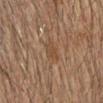Q: Was this lesion biopsied?
A: catalogued during a skin exam; not biopsied
Q: What are the patient's age and sex?
A: male, in their mid- to late 70s
Q: What is the imaging modality?
A: total-body-photography crop, ~15 mm field of view
Q: Illumination type?
A: cross-polarized illumination
Q: Automated lesion metrics?
A: a lesion area of about 3.5 mm², an outline eccentricity of about 0.9 (0 = round, 1 = elongated), and a shape-asymmetry score of about 0.25 (0 = symmetric); a mean CIELAB color near L≈37 a*≈15 b*≈26 and roughly 5 lightness units darker than nearby skin; a border-irregularity rating of about 3/10 and a color-variation rating of about 1.5/10
Q: What is the lesion's diameter?
A: ≈3 mm
Q: Lesion location?
A: the head or neck